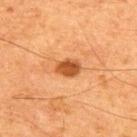{"biopsy_status": "not biopsied; imaged during a skin examination", "lesion_size": {"long_diameter_mm_approx": 3.0}, "image": {"source": "total-body photography crop", "field_of_view_mm": 15}, "patient": {"sex": "male", "age_approx": 65}, "automated_metrics": {"cielab_L": 44, "cielab_a": 26, "cielab_b": 38, "vs_skin_darker_L": 13.0, "vs_skin_contrast_norm": 10.0, "border_irregularity_0_10": 2.0, "color_variation_0_10": 3.0, "peripheral_color_asymmetry": 1.0, "nevus_likeness_0_100": 95, "lesion_detection_confidence_0_100": 100}, "site": "back", "lighting": "cross-polarized"}